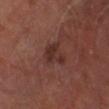{
  "biopsy_status": "not biopsied; imaged during a skin examination",
  "site": "right lower leg",
  "patient": {
    "sex": "male",
    "age_approx": 65
  },
  "lighting": "cross-polarized",
  "image": {
    "source": "total-body photography crop",
    "field_of_view_mm": 15
  }
}